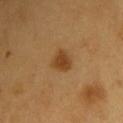Impression:
Recorded during total-body skin imaging; not selected for excision or biopsy.
Acquisition and patient details:
About 2.5 mm across. The subject is a male in their 60s. Captured under cross-polarized illumination. From the back. A 15 mm crop from a total-body photograph taken for skin-cancer surveillance.